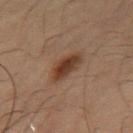This lesion was catalogued during total-body skin photography and was not selected for biopsy. A close-up tile cropped from a whole-body skin photograph, about 15 mm across. The lesion-visualizer software estimated a lesion–skin lightness drop of about 9 and a lesion-to-skin contrast of about 9 (normalized; higher = more distinct). The analysis additionally found a color-variation rating of about 5/10 and radial color variation of about 1.5. A male subject roughly 50 years of age. The lesion is on the abdomen. Longest diameter approximately 3.5 mm. Imaged with cross-polarized lighting.